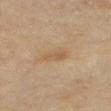Assessment:
Recorded during total-body skin imaging; not selected for excision or biopsy.
Clinical summary:
The total-body-photography lesion software estimated an outline eccentricity of about 0.9 (0 = round, 1 = elongated) and two-axis asymmetry of about 0.35. It also reported an average lesion color of about L≈48 a*≈13 b*≈31 (CIELAB), about 6 CIELAB-L* units darker than the surrounding skin, and a normalized border contrast of about 5.5. And it measured border irregularity of about 4 on a 0–10 scale, a within-lesion color-variation index near 1/10, and radial color variation of about 0.5. And it measured an automated nevus-likeness rating near 5 out of 100. A female patient aged 78 to 82. A lesion tile, about 15 mm wide, cut from a 3D total-body photograph. The lesion's longest dimension is about 3.5 mm. On the leg.